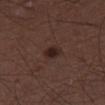- notes · imaged on a skin check; not biopsied
- size · ~2.5 mm (longest diameter)
- image-analysis metrics · an eccentricity of roughly 0.8; about 9 CIELAB-L* units darker than the surrounding skin and a normalized border contrast of about 9.5
- location · the right thigh
- image · ~15 mm crop, total-body skin-cancer survey
- subject · male, aged approximately 50
- illumination · white-light illumination